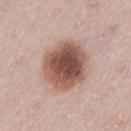Recorded during total-body skin imaging; not selected for excision or biopsy. A female subject, roughly 30 years of age. Automated image analysis of the tile measured an average lesion color of about L≈52 a*≈22 b*≈25 (CIELAB). It also reported border irregularity of about 1 on a 0–10 scale, a within-lesion color-variation index near 7/10, and peripheral color asymmetry of about 2. The software also gave a lesion-detection confidence of about 100/100. A close-up tile cropped from a whole-body skin photograph, about 15 mm across. The tile uses white-light illumination. Measured at roughly 5.5 mm in maximum diameter. The lesion is on the lower back.